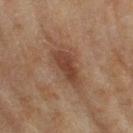Imaged during a routine full-body skin examination; the lesion was not biopsied and no histopathology is available.
This is a cross-polarized tile.
A 15 mm close-up extracted from a 3D total-body photography capture.
A female subject aged 58 to 62.
Located on the leg.